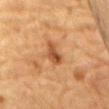workup — catalogued during a skin exam; not biopsied | acquisition — 15 mm crop, total-body photography | subject — male, aged 83 or older | site — the chest | lesion diameter — ~3.5 mm (longest diameter).